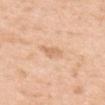Assessment: No biopsy was performed on this lesion — it was imaged during a full skin examination and was not determined to be concerning. Acquisition and patient details: A female patient in their 40s. A 15 mm close-up extracted from a 3D total-body photography capture. Measured at roughly 3 mm in maximum diameter. Automated tile analysis of the lesion measured a mean CIELAB color near L≈69 a*≈21 b*≈36 and a lesion–skin lightness drop of about 8. It also reported border irregularity of about 3 on a 0–10 scale, a color-variation rating of about 1/10, and radial color variation of about 0.5. The software also gave an automated nevus-likeness rating near 0 out of 100 and a detector confidence of about 100 out of 100 that the crop contains a lesion. The lesion is located on the back. Captured under white-light illumination.